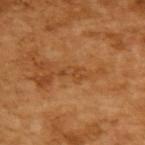This lesion was catalogued during total-body skin photography and was not selected for biopsy. The lesion-visualizer software estimated an average lesion color of about L≈44 a*≈23 b*≈39 (CIELAB), a lesion–skin lightness drop of about 7, and a lesion-to-skin contrast of about 5.5 (normalized; higher = more distinct). The analysis additionally found a nevus-likeness score of about 0/100 and a detector confidence of about 95 out of 100 that the crop contains a lesion. A 15 mm crop from a total-body photograph taken for skin-cancer surveillance. The patient is a male aged 63–67. Measured at roughly 3 mm in maximum diameter.